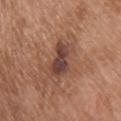<record>
  <biopsy_status>not biopsied; imaged during a skin examination</biopsy_status>
  <image>
    <source>total-body photography crop</source>
    <field_of_view_mm>15</field_of_view_mm>
  </image>
  <patient>
    <sex>male</sex>
    <age_approx>65</age_approx>
  </patient>
  <site>chest</site>
</record>